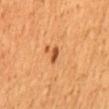follow-up=catalogued during a skin exam; not biopsied | diameter=~2.5 mm (longest diameter) | subject=male, in their mid-40s | image=~15 mm crop, total-body skin-cancer survey | location=the mid back | automated metrics=an average lesion color of about L≈50 a*≈27 b*≈42 (CIELAB), roughly 13 lightness units darker than nearby skin, and a normalized lesion–skin contrast near 9; a border-irregularity rating of about 2.5/10, internal color variation of about 0 on a 0–10 scale, and peripheral color asymmetry of about 0 | tile lighting=cross-polarized illumination.